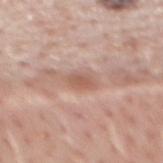<lesion>
<biopsy_status>not biopsied; imaged during a skin examination</biopsy_status>
<image>
  <source>total-body photography crop</source>
  <field_of_view_mm>15</field_of_view_mm>
</image>
<lesion_size>
  <long_diameter_mm_approx>3.0</long_diameter_mm_approx>
</lesion_size>
<site>mid back</site>
<patient>
  <sex>male</sex>
  <age_approx>60</age_approx>
</patient>
<lighting>white-light</lighting>
</lesion>